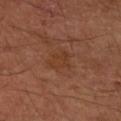| feature | finding |
|---|---|
| biopsy status | catalogued during a skin exam; not biopsied |
| image source | total-body-photography crop, ~15 mm field of view |
| lesion size | ≈3.5 mm |
| subject | male, approximately 50 years of age |
| tile lighting | cross-polarized illumination |
| body site | the arm |